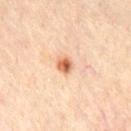Captured during whole-body skin photography for melanoma surveillance; the lesion was not biopsied. On the front of the torso. The patient is a male about 35 years old. A 15 mm close-up tile from a total-body photography series done for melanoma screening. Measured at roughly 2 mm in maximum diameter. Automated tile analysis of the lesion measured a border-irregularity index near 2/10 and a peripheral color-asymmetry measure near 2. The software also gave a nevus-likeness score of about 95/100.